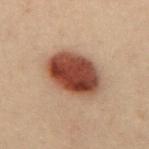image source: total-body-photography crop, ~15 mm field of view
site: the mid back
subject: female, in their 30s